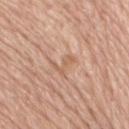Clinical impression:
Captured during whole-body skin photography for melanoma surveillance; the lesion was not biopsied.
Acquisition and patient details:
The lesion is on the right thigh. A 15 mm close-up tile from a total-body photography series done for melanoma screening. A female subject, aged 73 to 77.